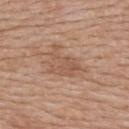{
  "biopsy_status": "not biopsied; imaged during a skin examination",
  "lighting": "white-light",
  "automated_metrics": {
    "area_mm2_approx": 10.0,
    "eccentricity": 0.75,
    "shape_asymmetry": 0.45,
    "border_irregularity_0_10": 6.0,
    "color_variation_0_10": 3.0,
    "nevus_likeness_0_100": 0,
    "lesion_detection_confidence_0_100": 100
  },
  "image": {
    "source": "total-body photography crop",
    "field_of_view_mm": 15
  },
  "patient": {
    "sex": "female",
    "age_approx": 50
  },
  "lesion_size": {
    "long_diameter_mm_approx": 5.0
  },
  "site": "upper back"
}